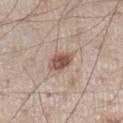No biopsy was performed on this lesion — it was imaged during a full skin examination and was not determined to be concerning.
The tile uses white-light illumination.
This image is a 15 mm lesion crop taken from a total-body photograph.
A male subject roughly 60 years of age.
Longest diameter approximately 3 mm.
From the left lower leg.